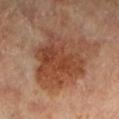Part of a total-body skin-imaging series; this lesion was reviewed on a skin check and was not flagged for biopsy.
Measured at roughly 8.5 mm in maximum diameter.
The patient is a female in their 70s.
A lesion tile, about 15 mm wide, cut from a 3D total-body photograph.
The lesion is located on the left lower leg.
The tile uses cross-polarized illumination.